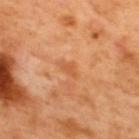follow-up: imaged on a skin check; not biopsied